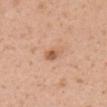notes: imaged on a skin check; not biopsied | automated metrics: an outline eccentricity of about 0.75 (0 = round, 1 = elongated); a lesion color around L≈60 a*≈22 b*≈33 in CIELAB, a lesion–skin lightness drop of about 10, and a normalized border contrast of about 6.5; a border-irregularity index near 2.5/10 and peripheral color asymmetry of about 2; an automated nevus-likeness rating near 55 out of 100 | illumination: white-light | site: the left upper arm | patient: female, aged around 30 | lesion size: about 3 mm | image: ~15 mm tile from a whole-body skin photo.